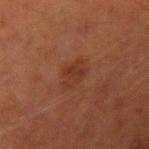The lesion was photographed on a routine skin check and not biopsied; there is no pathology result. Captured under cross-polarized illumination. On the right upper arm. A male patient about 45 years old. A region of skin cropped from a whole-body photographic capture, roughly 15 mm wide. The total-body-photography lesion software estimated a border-irregularity index near 3/10, a within-lesion color-variation index near 2/10, and peripheral color asymmetry of about 0.5. The software also gave a classifier nevus-likeness of about 10/100 and a detector confidence of about 100 out of 100 that the crop contains a lesion. About 3 mm across.